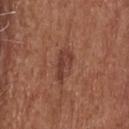Q: Was a biopsy performed?
A: no biopsy performed (imaged during a skin exam)
Q: What lighting was used for the tile?
A: white-light
Q: Who is the patient?
A: male, approximately 75 years of age
Q: What kind of image is this?
A: ~15 mm tile from a whole-body skin photo
Q: What is the anatomic site?
A: the head or neck
Q: Automated lesion metrics?
A: a footprint of about 6.5 mm², a shape eccentricity near 0.9, and a shape-asymmetry score of about 0.3 (0 = symmetric); a mean CIELAB color near L≈40 a*≈23 b*≈27, roughly 8 lightness units darker than nearby skin, and a lesion-to-skin contrast of about 7 (normalized; higher = more distinct); an automated nevus-likeness rating near 35 out of 100 and lesion-presence confidence of about 100/100